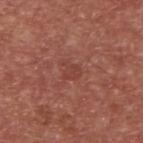The lesion was photographed on a routine skin check and not biopsied; there is no pathology result. Located on the upper back. A male patient, aged 63–67. A 15 mm close-up extracted from a 3D total-body photography capture.